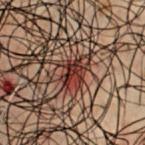Recorded during total-body skin imaging; not selected for excision or biopsy.
The tile uses cross-polarized illumination.
On the chest.
A male subject, aged around 50.
An algorithmic analysis of the crop reported an area of roughly 4.5 mm², a shape eccentricity near 0.7, and a symmetry-axis asymmetry near 0.35. And it measured a mean CIELAB color near L≈22 a*≈20 b*≈19 and a lesion–skin lightness drop of about 11. And it measured a nevus-likeness score of about 75/100 and a detector confidence of about 100 out of 100 that the crop contains a lesion.
Measured at roughly 2.5 mm in maximum diameter.
A 15 mm close-up extracted from a 3D total-body photography capture.